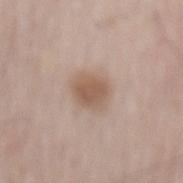This lesion was catalogued during total-body skin photography and was not selected for biopsy. The tile uses white-light illumination. About 3.5 mm across. The lesion is on the mid back. A male subject, aged 63 to 67. A 15 mm close-up tile from a total-body photography series done for melanoma screening.